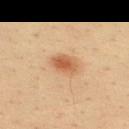This lesion was catalogued during total-body skin photography and was not selected for biopsy. This image is a 15 mm lesion crop taken from a total-body photograph. On the upper back. The subject is a male about 35 years old. Imaged with cross-polarized lighting.